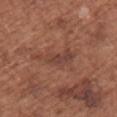workup — imaged on a skin check; not biopsied | subject — female, aged 73–77 | site — the upper back | acquisition — 15 mm crop, total-body photography.